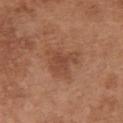| key | value |
|---|---|
| workup | catalogued during a skin exam; not biopsied |
| lesion size | ≈4 mm |
| image source | total-body-photography crop, ~15 mm field of view |
| subject | female, in their mid-60s |
| automated lesion analysis | a lesion area of about 9 mm², an outline eccentricity of about 0.5 (0 = round, 1 = elongated), and a symmetry-axis asymmetry near 0.45; a lesion color around L≈46 a*≈23 b*≈31 in CIELAB, about 8 CIELAB-L* units darker than the surrounding skin, and a normalized border contrast of about 6 |
| tile lighting | white-light illumination |
| location | the chest |